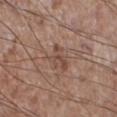Q: Was this lesion biopsied?
A: no biopsy performed (imaged during a skin exam)
Q: Where on the body is the lesion?
A: the right lower leg
Q: What lighting was used for the tile?
A: white-light
Q: Patient demographics?
A: male, roughly 65 years of age
Q: What is the imaging modality?
A: ~15 mm tile from a whole-body skin photo
Q: Automated lesion metrics?
A: a border-irregularity index near 4.5/10 and peripheral color asymmetry of about 1.5; a classifier nevus-likeness of about 0/100 and a detector confidence of about 100 out of 100 that the crop contains a lesion